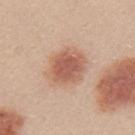The tile uses white-light illumination. A lesion tile, about 15 mm wide, cut from a 3D total-body photograph. A female subject, aged approximately 25. About 4.5 mm across. On the left upper arm. Automated image analysis of the tile measured a border-irregularity index near 1.5/10 and radial color variation of about 1. The software also gave a nevus-likeness score of about 100/100 and lesion-presence confidence of about 100/100.